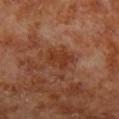| key | value |
|---|---|
| patient | male, roughly 70 years of age |
| imaging modality | ~15 mm tile from a whole-body skin photo |
| lighting | cross-polarized |
| site | the left lower leg |
| lesion size | ~3 mm (longest diameter) |